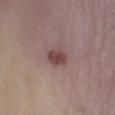| key | value |
|---|---|
| imaging modality | ~15 mm crop, total-body skin-cancer survey |
| patient | female, in their mid-30s |
| lesion diameter | about 2.5 mm |
| site | the left leg |
| tile lighting | white-light |